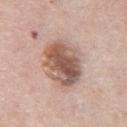Q: Is there a histopathology result?
A: no biopsy performed (imaged during a skin exam)
Q: How was this image acquired?
A: ~15 mm tile from a whole-body skin photo
Q: Who is the patient?
A: male, in their mid-70s
Q: Illumination type?
A: white-light
Q: Lesion size?
A: about 5.5 mm
Q: Where on the body is the lesion?
A: the abdomen